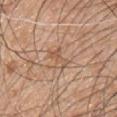| feature | finding |
|---|---|
| workup | imaged on a skin check; not biopsied |
| tile lighting | white-light illumination |
| automated lesion analysis | a footprint of about 5 mm², an eccentricity of roughly 0.7, and two-axis asymmetry of about 0.35; an average lesion color of about L≈56 a*≈19 b*≈31 (CIELAB), a lesion–skin lightness drop of about 7, and a normalized lesion–skin contrast near 5.5; border irregularity of about 4.5 on a 0–10 scale, a within-lesion color-variation index near 4/10, and peripheral color asymmetry of about 1.5; an automated nevus-likeness rating near 0 out of 100 and a detector confidence of about 100 out of 100 that the crop contains a lesion |
| location | the chest |
| subject | male, in their 50s |
| image | ~15 mm tile from a whole-body skin photo |